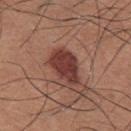No biopsy was performed on this lesion — it was imaged during a full skin examination and was not determined to be concerning. A 15 mm close-up tile from a total-body photography series done for melanoma screening. The lesion is on the chest. Longest diameter approximately 4.5 mm. This is a white-light tile. A male subject, aged around 35.